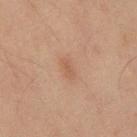  biopsy_status: not biopsied; imaged during a skin examination
  patient:
    sex: male
    age_approx: 45
  lesion_size:
    long_diameter_mm_approx: 2.5
  site: mid back
  image:
    source: total-body photography crop
    field_of_view_mm: 15